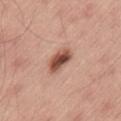Impression: The lesion was tiled from a total-body skin photograph and was not biopsied.